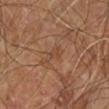TBP lesion metrics — a lesion color around L≈42 a*≈20 b*≈29 in CIELAB, a lesion–skin lightness drop of about 5, and a lesion-to-skin contrast of about 4.5 (normalized; higher = more distinct) | imaging modality — total-body-photography crop, ~15 mm field of view | lighting — cross-polarized | lesion size — about 3 mm | anatomic site — the right leg | subject — male, approximately 60 years of age.